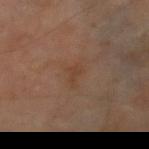Captured during whole-body skin photography for melanoma surveillance; the lesion was not biopsied. An algorithmic analysis of the crop reported a border-irregularity rating of about 4.5/10 and a peripheral color-asymmetry measure near 0. The analysis additionally found a classifier nevus-likeness of about 0/100. The subject is a male about 70 years old. The recorded lesion diameter is about 2.5 mm. A roughly 15 mm field-of-view crop from a total-body skin photograph. From the right thigh.